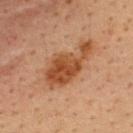Impression: The lesion was photographed on a routine skin check and not biopsied; there is no pathology result. Context: Captured under cross-polarized illumination. A male subject, in their mid-30s. The recorded lesion diameter is about 7 mm. A 15 mm close-up extracted from a 3D total-body photography capture. The lesion is on the upper back.